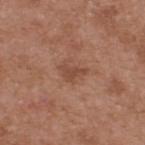Impression: The lesion was tiled from a total-body skin photograph and was not biopsied. Context: From the upper back. A male subject, aged around 55. A 15 mm close-up extracted from a 3D total-body photography capture.